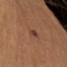Impression: Captured during whole-body skin photography for melanoma surveillance; the lesion was not biopsied.